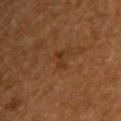  biopsy_status: not biopsied; imaged during a skin examination
  image:
    source: total-body photography crop
    field_of_view_mm: 15
  patient:
    sex: male
    age_approx: 65
  site: back
  lesion_size:
    long_diameter_mm_approx: 2.5
  automated_metrics:
    nevus_likeness_0_100: 0